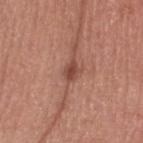Cropped from a whole-body photographic skin survey; the tile spans about 15 mm.
Located on the head or neck.
The subject is a male in their 30s.